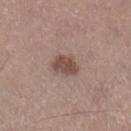{
  "biopsy_status": "not biopsied; imaged during a skin examination",
  "lesion_size": {
    "long_diameter_mm_approx": 3.5
  },
  "site": "leg",
  "lighting": "white-light",
  "image": {
    "source": "total-body photography crop",
    "field_of_view_mm": 15
  },
  "automated_metrics": {
    "eccentricity": 0.7,
    "vs_skin_darker_L": 11.0,
    "border_irregularity_0_10": 2.0,
    "color_variation_0_10": 3.0,
    "peripheral_color_asymmetry": 1.0,
    "nevus_likeness_0_100": 50
  },
  "patient": {
    "sex": "male",
    "age_approx": 45
  }
}